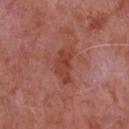  biopsy_status: not biopsied; imaged during a skin examination
  patient:
    sex: male
    age_approx: 65
  site: chest
  image:
    source: total-body photography crop
    field_of_view_mm: 15